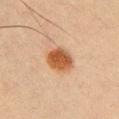  biopsy_status: not biopsied; imaged during a skin examination
  lighting: cross-polarized
  patient:
    sex: male
    age_approx: 60
  lesion_size:
    long_diameter_mm_approx: 4.0
  image:
    source: total-body photography crop
    field_of_view_mm: 15
  site: chest